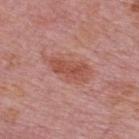<record>
  <biopsy_status>not biopsied; imaged during a skin examination</biopsy_status>
  <patient>
    <sex>male</sex>
    <age_approx>75</age_approx>
  </patient>
  <image>
    <source>total-body photography crop</source>
    <field_of_view_mm>15</field_of_view_mm>
  </image>
  <lighting>white-light</lighting>
  <automated_metrics>
    <eccentricity>0.9</eccentricity>
    <shape_asymmetry>0.35</shape_asymmetry>
    <nevus_likeness_0_100>25</nevus_likeness_0_100>
    <lesion_detection_confidence_0_100>100</lesion_detection_confidence_0_100>
  </automated_metrics>
  <site>upper back</site>
</record>